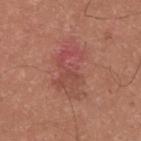Q: Was this lesion biopsied?
A: no biopsy performed (imaged during a skin exam)
Q: Patient demographics?
A: male, in their mid-20s
Q: Illumination type?
A: white-light
Q: How large is the lesion?
A: ≈7 mm
Q: What is the anatomic site?
A: the upper back
Q: What kind of image is this?
A: 15 mm crop, total-body photography